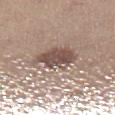workup = no biopsy performed (imaged during a skin exam)
illumination = white-light illumination
location = the left lower leg
patient = female, aged 43 to 47
diameter = ≈5 mm
image source = ~15 mm crop, total-body skin-cancer survey